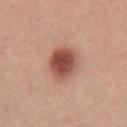notes: total-body-photography surveillance lesion; no biopsy | image: ~15 mm crop, total-body skin-cancer survey | tile lighting: white-light illumination | patient: female, aged around 40 | body site: the chest.